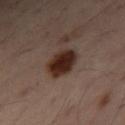{
  "patient": {
    "sex": "male",
    "age_approx": 50
  },
  "lesion_size": {
    "long_diameter_mm_approx": 4.0
  },
  "automated_metrics": {
    "area_mm2_approx": 9.5,
    "eccentricity": 0.7,
    "shape_asymmetry": 0.2,
    "cielab_L": 26,
    "cielab_a": 17,
    "cielab_b": 22,
    "vs_skin_darker_L": 15.0
  },
  "image": {
    "source": "total-body photography crop",
    "field_of_view_mm": 15
  },
  "site": "abdomen",
  "lighting": "cross-polarized"
}